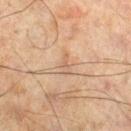Recorded during total-body skin imaging; not selected for excision or biopsy. The subject is a male in their mid-40s. This is a cross-polarized tile. On the right lower leg. A 15 mm close-up extracted from a 3D total-body photography capture. Measured at roughly 2.5 mm in maximum diameter. The lesion-visualizer software estimated a mean CIELAB color near L≈49 a*≈16 b*≈27. The software also gave a border-irregularity index near 5/10, internal color variation of about 0 on a 0–10 scale, and a peripheral color-asymmetry measure near 0. The analysis additionally found an automated nevus-likeness rating near 0 out of 100.